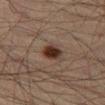workup: no biopsy performed (imaged during a skin exam) | automated lesion analysis: a lesion area of about 5.5 mm², a shape eccentricity near 0.65, and two-axis asymmetry of about 0.2; border irregularity of about 2 on a 0–10 scale, internal color variation of about 4.5 on a 0–10 scale, and radial color variation of about 1.5; a classifier nevus-likeness of about 100/100 and a detector confidence of about 100 out of 100 that the crop contains a lesion | tile lighting: cross-polarized illumination | size: about 3 mm | subject: male, roughly 50 years of age | image: total-body-photography crop, ~15 mm field of view | site: the left thigh.